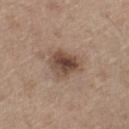follow-up = catalogued during a skin exam; not biopsied | image = total-body-photography crop, ~15 mm field of view | tile lighting = white-light illumination | subject = male, roughly 70 years of age | diameter = ≈4 mm | site = the left thigh.